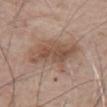This lesion was catalogued during total-body skin photography and was not selected for biopsy.
A 15 mm crop from a total-body photograph taken for skin-cancer surveillance.
The patient is a male approximately 65 years of age.
This is a white-light tile.
From the chest.
The recorded lesion diameter is about 5.5 mm.
Automated tile analysis of the lesion measured a lesion area of about 21 mm², an eccentricity of roughly 0.65, and a shape-asymmetry score of about 0.3 (0 = symmetric). The analysis additionally found a border-irregularity rating of about 4/10 and a peripheral color-asymmetry measure near 1.5. It also reported a nevus-likeness score of about 70/100 and a lesion-detection confidence of about 100/100.